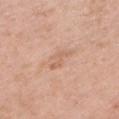<lesion>
<image>
  <source>total-body photography crop</source>
  <field_of_view_mm>15</field_of_view_mm>
</image>
<lesion_size>
  <long_diameter_mm_approx>3.5</long_diameter_mm_approx>
</lesion_size>
<patient>
  <sex>female</sex>
  <age_approx>70</age_approx>
</patient>
<site>left lower leg</site>
</lesion>